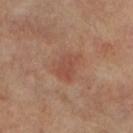workup — total-body-photography surveillance lesion; no biopsy
subject — female, in their mid-60s
site — the right leg
image source — ~15 mm crop, total-body skin-cancer survey